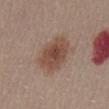notes: catalogued during a skin exam; not biopsied
subject: female, roughly 30 years of age
size: ~5.5 mm (longest diameter)
body site: the abdomen
image source: ~15 mm tile from a whole-body skin photo